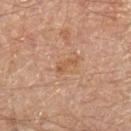follow-up = no biopsy performed (imaged during a skin exam) | size = about 3 mm | location = the left forearm | image = total-body-photography crop, ~15 mm field of view | subject = male, aged around 45.